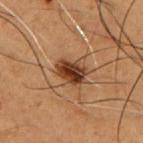Recorded during total-body skin imaging; not selected for excision or biopsy. The recorded lesion diameter is about 3.5 mm. From the chest. The patient is a male aged 48–52. The tile uses cross-polarized illumination. A roughly 15 mm field-of-view crop from a total-body skin photograph. Automated image analysis of the tile measured a border-irregularity rating of about 2/10, a color-variation rating of about 6.5/10, and peripheral color asymmetry of about 2.